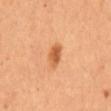{
  "biopsy_status": "not biopsied; imaged during a skin examination",
  "site": "abdomen",
  "automated_metrics": {
    "cielab_L": 60,
    "cielab_a": 28,
    "cielab_b": 43,
    "vs_skin_darker_L": 12.0,
    "vs_skin_contrast_norm": 8.0,
    "border_irregularity_0_10": 1.5,
    "color_variation_0_10": 3.5
  },
  "image": {
    "source": "total-body photography crop",
    "field_of_view_mm": 15
  },
  "lesion_size": {
    "long_diameter_mm_approx": 3.0
  },
  "patient": {
    "sex": "female"
  }
}